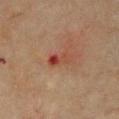follow-up: no biopsy performed (imaged during a skin exam)
subject: female, roughly 70 years of age
image source: ~15 mm tile from a whole-body skin photo
lesion size: ~3 mm (longest diameter)
location: the chest
tile lighting: cross-polarized illumination